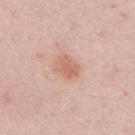The lesion was photographed on a routine skin check and not biopsied; there is no pathology result.
Captured under white-light illumination.
The subject is a male in their mid-20s.
The lesion's longest dimension is about 3 mm.
Automated tile analysis of the lesion measured an automated nevus-likeness rating near 65 out of 100 and a detector confidence of about 100 out of 100 that the crop contains a lesion.
On the back.
This image is a 15 mm lesion crop taken from a total-body photograph.